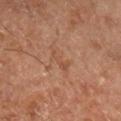Assessment: Imaged during a routine full-body skin examination; the lesion was not biopsied and no histopathology is available. Acquisition and patient details: The subject is roughly 65 years of age. Located on the right lower leg. Automated image analysis of the tile measured a lesion area of about 3 mm² and two-axis asymmetry of about 0.45. And it measured a lesion color around L≈49 a*≈22 b*≈32 in CIELAB, a lesion–skin lightness drop of about 6, and a lesion-to-skin contrast of about 5 (normalized; higher = more distinct). And it measured border irregularity of about 6 on a 0–10 scale and internal color variation of about 0 on a 0–10 scale. The software also gave an automated nevus-likeness rating near 0 out of 100. This image is a 15 mm lesion crop taken from a total-body photograph. About 3 mm across. The tile uses cross-polarized illumination.